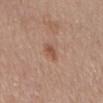| key | value |
|---|---|
| notes | catalogued during a skin exam; not biopsied |
| anatomic site | the front of the torso |
| patient | male, approximately 80 years of age |
| tile lighting | white-light illumination |
| image-analysis metrics | a lesion–skin lightness drop of about 9 and a normalized border contrast of about 7; border irregularity of about 2.5 on a 0–10 scale, internal color variation of about 1.5 on a 0–10 scale, and a peripheral color-asymmetry measure near 0.5; a nevus-likeness score of about 60/100 and a detector confidence of about 100 out of 100 that the crop contains a lesion |
| size | ≈2.5 mm |
| image | ~15 mm tile from a whole-body skin photo |